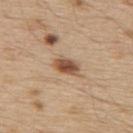The lesion was tiled from a total-body skin photograph and was not biopsied.
Imaged with white-light lighting.
A 15 mm close-up tile from a total-body photography series done for melanoma screening.
The lesion's longest dimension is about 3 mm.
A male patient, aged 68–72.
An algorithmic analysis of the crop reported a footprint of about 5 mm², an eccentricity of roughly 0.8, and a symmetry-axis asymmetry near 0.2. And it measured an average lesion color of about L≈52 a*≈19 b*≈32 (CIELAB) and about 15 CIELAB-L* units darker than the surrounding skin. It also reported a nevus-likeness score of about 95/100.
From the upper back.